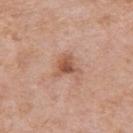A close-up tile cropped from a whole-body skin photograph, about 15 mm across.
A female subject aged 68 to 72.
Measured at roughly 3 mm in maximum diameter.
The lesion is on the right upper arm.